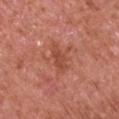No biopsy was performed on this lesion — it was imaged during a full skin examination and was not determined to be concerning.
Approximately 4 mm at its widest.
The subject is a male in their mid- to late 60s.
The lesion is on the arm.
The tile uses white-light illumination.
A close-up tile cropped from a whole-body skin photograph, about 15 mm across.